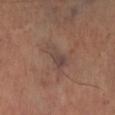Notes:
• biopsy status — imaged on a skin check; not biopsied
• image — ~15 mm tile from a whole-body skin photo
• lighting — cross-polarized illumination
• location — the left lower leg
• automated metrics — a lesion–skin lightness drop of about 6 and a lesion-to-skin contrast of about 6 (normalized; higher = more distinct); border irregularity of about 4.5 on a 0–10 scale, internal color variation of about 4 on a 0–10 scale, and a peripheral color-asymmetry measure near 1.5; a lesion-detection confidence of about 90/100